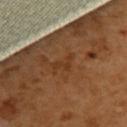Case summary:
* workup — total-body-photography surveillance lesion; no biopsy
* illumination — cross-polarized
* automated metrics — an area of roughly 2.5 mm², an outline eccentricity of about 0.85 (0 = round, 1 = elongated), and a shape-asymmetry score of about 0.75 (0 = symmetric); a lesion color around L≈36 a*≈22 b*≈34 in CIELAB and a lesion–skin lightness drop of about 5
* size — ~3 mm (longest diameter)
* imaging modality — total-body-photography crop, ~15 mm field of view
* site — the upper back
* subject — female, in their mid-50s